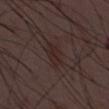Q: Was this lesion biopsied?
A: imaged on a skin check; not biopsied
Q: What did automated image analysis measure?
A: a normalized lesion–skin contrast near 6
Q: Patient demographics?
A: male, approximately 50 years of age
Q: What is the imaging modality?
A: total-body-photography crop, ~15 mm field of view
Q: What lighting was used for the tile?
A: white-light
Q: Lesion size?
A: ~3 mm (longest diameter)